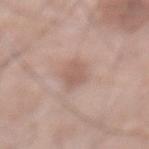  lesion_size:
    long_diameter_mm_approx: 3.0
  automated_metrics:
    cielab_L: 58
    cielab_a: 18
    cielab_b: 24
    vs_skin_darker_L: 8.0
    vs_skin_contrast_norm: 6.0
    border_irregularity_0_10: 3.0
    color_variation_0_10: 1.5
    peripheral_color_asymmetry: 0.5
    nevus_likeness_0_100: 20
  patient:
    sex: male
    age_approx: 70
  site: abdomen
  lighting: white-light
  image:
    source: total-body photography crop
    field_of_view_mm: 15